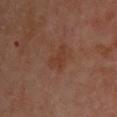The lesion was tiled from a total-body skin photograph and was not biopsied. The lesion is on the back. A male patient, about 50 years old. The total-body-photography lesion software estimated a footprint of about 5 mm², a shape eccentricity near 0.75, and a symmetry-axis asymmetry near 0.5. The software also gave border irregularity of about 4.5 on a 0–10 scale, a color-variation rating of about 1/10, and a peripheral color-asymmetry measure near 0.5. A close-up tile cropped from a whole-body skin photograph, about 15 mm across. Imaged with cross-polarized lighting.